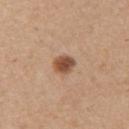A roughly 15 mm field-of-view crop from a total-body skin photograph. A female patient aged 43–47. The lesion's longest dimension is about 2.5 mm. The lesion is located on the left upper arm. Imaged with white-light lighting.